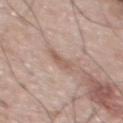notes: total-body-photography surveillance lesion; no biopsy | imaging modality: ~15 mm tile from a whole-body skin photo | anatomic site: the upper back | lighting: white-light illumination | automated metrics: about 8 CIELAB-L* units darker than the surrounding skin and a normalized lesion–skin contrast near 5.5; a border-irregularity rating of about 2.5/10, internal color variation of about 3 on a 0–10 scale, and peripheral color asymmetry of about 1 | patient: male, in their mid- to late 50s | size: about 3 mm.